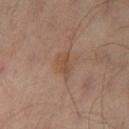  biopsy_status: not biopsied; imaged during a skin examination
  site: left thigh
  image:
    source: total-body photography crop
    field_of_view_mm: 15
  lesion_size:
    long_diameter_mm_approx: 2.5
  patient:
    sex: male
    age_approx: 65
  lighting: cross-polarized